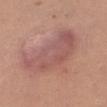acquisition=~15 mm crop, total-body skin-cancer survey | patient=female, aged approximately 30 | anatomic site=the leg.